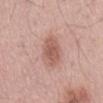Q: Was a biopsy performed?
A: imaged on a skin check; not biopsied
Q: What is the lesion's diameter?
A: ~5 mm (longest diameter)
Q: What is the imaging modality?
A: total-body-photography crop, ~15 mm field of view
Q: What are the patient's age and sex?
A: male, aged approximately 55
Q: What is the anatomic site?
A: the front of the torso
Q: How was the tile lit?
A: white-light illumination